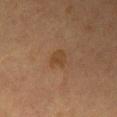From the mid back. A roughly 15 mm field-of-view crop from a total-body skin photograph. The patient is a male aged approximately 55.